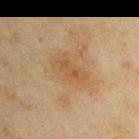The lesion was photographed on a routine skin check and not biopsied; there is no pathology result. Measured at roughly 4.5 mm in maximum diameter. The tile uses cross-polarized illumination. Located on the arm. Automated image analysis of the tile measured an outline eccentricity of about 0.85 (0 = round, 1 = elongated) and two-axis asymmetry of about 0.35. The software also gave an average lesion color of about L≈43 a*≈15 b*≈31 (CIELAB), roughly 6 lightness units darker than nearby skin, and a normalized lesion–skin contrast near 6. It also reported a within-lesion color-variation index near 2.5/10 and a peripheral color-asymmetry measure near 1. The software also gave a nevus-likeness score of about 0/100 and a detector confidence of about 100 out of 100 that the crop contains a lesion. The subject is a male in their mid- to late 40s. A 15 mm close-up tile from a total-body photography series done for melanoma screening.